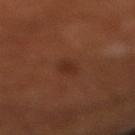| field | value |
|---|---|
| workup | catalogued during a skin exam; not biopsied |
| image | total-body-photography crop, ~15 mm field of view |
| patient | male, about 70 years old |
| body site | the right lower leg |
| image-analysis metrics | a border-irregularity rating of about 2.5/10, a color-variation rating of about 1.5/10, and a peripheral color-asymmetry measure near 0.5 |
| lesion diameter | ~2.5 mm (longest diameter) |
| illumination | cross-polarized illumination |